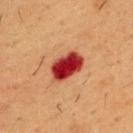Q: Was this lesion biopsied?
A: catalogued during a skin exam; not biopsied
Q: What did automated image analysis measure?
A: peripheral color asymmetry of about 1.5; a nevus-likeness score of about 0/100 and lesion-presence confidence of about 100/100
Q: Lesion location?
A: the chest
Q: How large is the lesion?
A: ~4.5 mm (longest diameter)
Q: How was this image acquired?
A: total-body-photography crop, ~15 mm field of view
Q: What are the patient's age and sex?
A: male, aged around 55